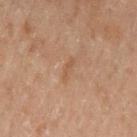Assessment: Recorded during total-body skin imaging; not selected for excision or biopsy. Image and clinical context: A male subject, aged 68–72. Cropped from a whole-body photographic skin survey; the tile spans about 15 mm. The recorded lesion diameter is about 3 mm. Imaged with cross-polarized lighting. An algorithmic analysis of the crop reported an area of roughly 2.5 mm², an eccentricity of roughly 0.95, and two-axis asymmetry of about 0.4. It also reported a lesion color around L≈40 a*≈15 b*≈26 in CIELAB and about 4 CIELAB-L* units darker than the surrounding skin. And it measured border irregularity of about 4.5 on a 0–10 scale and a peripheral color-asymmetry measure near 0. The analysis additionally found a detector confidence of about 100 out of 100 that the crop contains a lesion. From the lower back.